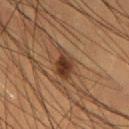The lesion was tiled from a total-body skin photograph and was not biopsied.
Longest diameter approximately 4 mm.
A male patient, aged 48–52.
Cropped from a whole-body photographic skin survey; the tile spans about 15 mm.
On the left thigh.
This is a cross-polarized tile.